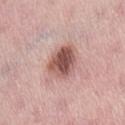Q: Was a biopsy performed?
A: catalogued during a skin exam; not biopsied
Q: Illumination type?
A: white-light illumination
Q: Lesion location?
A: the lower back
Q: How large is the lesion?
A: ~4 mm (longest diameter)
Q: Automated lesion metrics?
A: a footprint of about 11 mm² and an eccentricity of roughly 0.55; a mean CIELAB color near L≈54 a*≈23 b*≈24, a lesion–skin lightness drop of about 16, and a normalized border contrast of about 10; a border-irregularity rating of about 1.5/10, internal color variation of about 6 on a 0–10 scale, and a peripheral color-asymmetry measure near 2
Q: How was this image acquired?
A: ~15 mm tile from a whole-body skin photo
Q: Who is the patient?
A: female, aged around 65